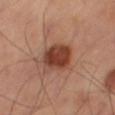– follow-up: catalogued during a skin exam; not biopsied
– illumination: cross-polarized
– site: the leg
– lesion diameter: ≈4 mm
– image source: 15 mm crop, total-body photography
– automated metrics: a mean CIELAB color near L≈42 a*≈26 b*≈30, about 14 CIELAB-L* units darker than the surrounding skin, and a normalized border contrast of about 10.5; border irregularity of about 1.5 on a 0–10 scale and a within-lesion color-variation index near 4/10; a classifier nevus-likeness of about 100/100 and a detector confidence of about 100 out of 100 that the crop contains a lesion